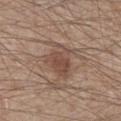Q: Was a biopsy performed?
A: imaged on a skin check; not biopsied
Q: Who is the patient?
A: male, aged approximately 80
Q: How was the tile lit?
A: white-light illumination
Q: Where on the body is the lesion?
A: the chest
Q: How large is the lesion?
A: ≈4.5 mm
Q: What did automated image analysis measure?
A: roughly 9 lightness units darker than nearby skin; a border-irregularity index near 4/10 and internal color variation of about 3.5 on a 0–10 scale
Q: What is the imaging modality?
A: ~15 mm crop, total-body skin-cancer survey